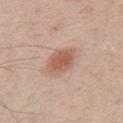The lesion was tiled from a total-body skin photograph and was not biopsied.
Approximately 4 mm at its widest.
The subject is a male aged 38 to 42.
The lesion is on the chest.
A lesion tile, about 15 mm wide, cut from a 3D total-body photograph.
This is a white-light tile.